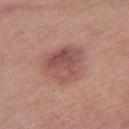Clinical summary: The subject is a female about 65 years old. On the right thigh. The recorded lesion diameter is about 5.5 mm. The total-body-photography lesion software estimated a lesion color around L≈52 a*≈23 b*≈23 in CIELAB, roughly 10 lightness units darker than nearby skin, and a normalized border contrast of about 7. A 15 mm crop from a total-body photograph taken for skin-cancer surveillance. The tile uses white-light illumination.